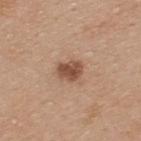workup: imaged on a skin check; not biopsied
imaging modality: total-body-photography crop, ~15 mm field of view
tile lighting: white-light illumination
anatomic site: the upper back
patient: male, aged 33 to 37
lesion diameter: ≈3 mm